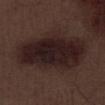This lesion was catalogued during total-body skin photography and was not selected for biopsy.
The lesion-visualizer software estimated a border-irregularity index near 2.5/10, a within-lesion color-variation index near 4.5/10, and radial color variation of about 1.5.
A male subject about 70 years old.
The tile uses white-light illumination.
Located on the left lower leg.
This image is a 15 mm lesion crop taken from a total-body photograph.